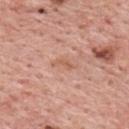This lesion was catalogued during total-body skin photography and was not selected for biopsy. The patient is a male in their 60s. A region of skin cropped from a whole-body photographic capture, roughly 15 mm wide. Imaged with white-light lighting. From the upper back. Automated tile analysis of the lesion measured an average lesion color of about L≈59 a*≈23 b*≈32 (CIELAB) and about 7 CIELAB-L* units darker than the surrounding skin. And it measured an automated nevus-likeness rating near 0 out of 100. Measured at roughly 3 mm in maximum diameter.